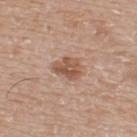notes: imaged on a skin check; not biopsied
imaging modality: ~15 mm crop, total-body skin-cancer survey
lighting: white-light illumination
body site: the upper back
subject: male, in their mid- to late 70s
TBP lesion metrics: a footprint of about 6 mm², a shape eccentricity near 0.65, and a shape-asymmetry score of about 0.3 (0 = symmetric); roughly 11 lightness units darker than nearby skin and a normalized border contrast of about 8; a detector confidence of about 100 out of 100 that the crop contains a lesion